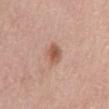No biopsy was performed on this lesion — it was imaged during a full skin examination and was not determined to be concerning. Located on the abdomen. A close-up tile cropped from a whole-body skin photograph, about 15 mm across. A male patient, aged approximately 80. Imaged with white-light lighting. Approximately 3 mm at its widest.